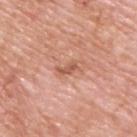{
  "biopsy_status": "not biopsied; imaged during a skin examination",
  "image": {
    "source": "total-body photography crop",
    "field_of_view_mm": 15
  },
  "lighting": "white-light",
  "patient": {
    "sex": "male",
    "age_approx": 60
  },
  "automated_metrics": {
    "cielab_L": 57,
    "cielab_a": 25,
    "cielab_b": 32,
    "vs_skin_darker_L": 9.0,
    "vs_skin_contrast_norm": 6.5
  },
  "site": "upper back"
}